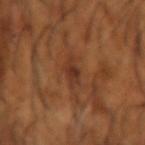biopsy status=imaged on a skin check; not biopsied
lesion size=≈3 mm
acquisition=total-body-photography crop, ~15 mm field of view
tile lighting=cross-polarized illumination
body site=the left forearm
subject=male, approximately 65 years of age